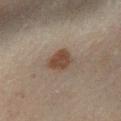<case>
  <biopsy_status>not biopsied; imaged during a skin examination</biopsy_status>
  <patient>
    <sex>male</sex>
    <age_approx>45</age_approx>
  </patient>
  <site>left lower leg</site>
  <lesion_size>
    <long_diameter_mm_approx>3.5</long_diameter_mm_approx>
  </lesion_size>
  <lighting>cross-polarized</lighting>
  <image>
    <source>total-body photography crop</source>
    <field_of_view_mm>15</field_of_view_mm>
  </image>
</case>